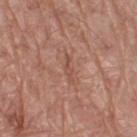| field | value |
|---|---|
| notes | catalogued during a skin exam; not biopsied |
| lesion diameter | about 3 mm |
| patient | male, roughly 70 years of age |
| body site | the right thigh |
| automated lesion analysis | an area of roughly 2 mm², an outline eccentricity of about 0.95 (0 = round, 1 = elongated), and a symmetry-axis asymmetry near 0.4; a mean CIELAB color near L≈50 a*≈24 b*≈27, roughly 7 lightness units darker than nearby skin, and a normalized lesion–skin contrast near 5.5 |
| image source | ~15 mm crop, total-body skin-cancer survey |
| illumination | white-light illumination |